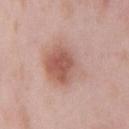biopsy status = imaged on a skin check; not biopsied
tile lighting = white-light illumination
diameter = ~7 mm (longest diameter)
imaging modality = 15 mm crop, total-body photography
TBP lesion metrics = a border-irregularity rating of about 2.5/10 and a within-lesion color-variation index near 7.5/10; a classifier nevus-likeness of about 95/100 and a lesion-detection confidence of about 100/100
body site = the chest
patient = male, aged 53 to 57